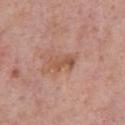– notes — total-body-photography surveillance lesion; no biopsy
– acquisition — ~15 mm crop, total-body skin-cancer survey
– automated metrics — a lesion area of about 4.5 mm², a shape eccentricity near 0.9, and two-axis asymmetry of about 0.3; an automated nevus-likeness rating near 0 out of 100 and a lesion-detection confidence of about 100/100
– tile lighting — white-light
– site — the chest
– patient — male, about 75 years old
– lesion diameter — ~3.5 mm (longest diameter)